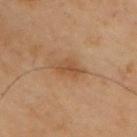Findings:
- workup: no biopsy performed (imaged during a skin exam)
- image-analysis metrics: a footprint of about 5.5 mm², an outline eccentricity of about 0.75 (0 = round, 1 = elongated), and a shape-asymmetry score of about 0.25 (0 = symmetric); a mean CIELAB color near L≈40 a*≈16 b*≈29 and a lesion–skin lightness drop of about 7
- patient: male, aged around 55
- imaging modality: ~15 mm tile from a whole-body skin photo
- size: about 3.5 mm
- body site: the chest